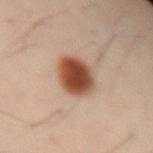Q: Is there a histopathology result?
A: catalogued during a skin exam; not biopsied
Q: What is the imaging modality?
A: total-body-photography crop, ~15 mm field of view
Q: Where on the body is the lesion?
A: the left forearm
Q: Who is the patient?
A: male, aged around 40